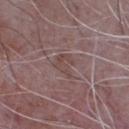Impression:
Recorded during total-body skin imaging; not selected for excision or biopsy.
Clinical summary:
The lesion is on the chest. The subject is a male aged 63–67. Cropped from a total-body skin-imaging series; the visible field is about 15 mm.